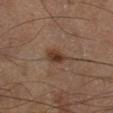workup = no biopsy performed (imaged during a skin exam); anatomic site = the right lower leg; patient = male, approximately 60 years of age; image = total-body-photography crop, ~15 mm field of view.